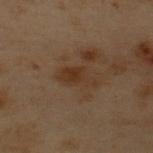| key | value |
|---|---|
| follow-up | catalogued during a skin exam; not biopsied |
| imaging modality | total-body-photography crop, ~15 mm field of view |
| tile lighting | cross-polarized illumination |
| lesion size | about 4 mm |
| patient | male, roughly 55 years of age |
| TBP lesion metrics | a footprint of about 7 mm²; a lesion color around L≈26 a*≈14 b*≈24 in CIELAB and a normalized border contrast of about 7; lesion-presence confidence of about 100/100 |
| site | the back |